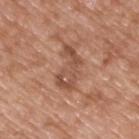Imaged during a routine full-body skin examination; the lesion was not biopsied and no histopathology is available.
About 5 mm across.
A male subject aged 48–52.
A lesion tile, about 15 mm wide, cut from a 3D total-body photograph.
The lesion is located on the upper back.
This is a white-light tile.